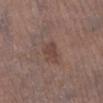Findings:
* workup — imaged on a skin check; not biopsied
* site — the leg
* subject — male, approximately 70 years of age
* lighting — white-light
* imaging modality — ~15 mm crop, total-body skin-cancer survey
* lesion diameter — about 2.5 mm